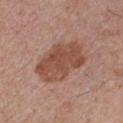Assessment: Imaged during a routine full-body skin examination; the lesion was not biopsied and no histopathology is available. Image and clinical context: Captured under white-light illumination. Longest diameter approximately 7 mm. A 15 mm close-up extracted from a 3D total-body photography capture. A male patient, aged around 30. From the chest.